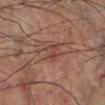Assessment:
Imaged during a routine full-body skin examination; the lesion was not biopsied and no histopathology is available.
Clinical summary:
An algorithmic analysis of the crop reported an area of roughly 4.5 mm² and a shape-asymmetry score of about 0.3 (0 = symmetric). And it measured lesion-presence confidence of about 90/100. A 15 mm close-up extracted from a 3D total-body photography capture. Located on the right lower leg.